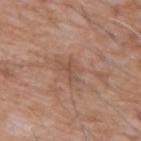automated lesion analysis: a color-variation rating of about 0/10; a nevus-likeness score of about 0/100 and lesion-presence confidence of about 95/100 | anatomic site: the chest | patient: male, in their 60s | size: ~2.5 mm (longest diameter) | illumination: white-light illumination | imaging modality: total-body-photography crop, ~15 mm field of view.